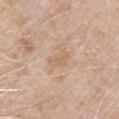{"biopsy_status": "not biopsied; imaged during a skin examination", "lesion_size": {"long_diameter_mm_approx": 3.0}, "image": {"source": "total-body photography crop", "field_of_view_mm": 15}, "patient": {"sex": "male", "age_approx": 80}, "lighting": "white-light", "site": "right upper arm", "automated_metrics": {"area_mm2_approx": 4.5, "eccentricity": 0.75, "cielab_L": 64, "cielab_a": 17, "cielab_b": 31, "vs_skin_darker_L": 6.0, "vs_skin_contrast_norm": 4.5, "border_irregularity_0_10": 3.5, "color_variation_0_10": 1.5}}